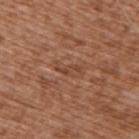follow-up — catalogued during a skin exam; not biopsied | illumination — white-light illumination | automated lesion analysis — a shape eccentricity near 0.95 and a shape-asymmetry score of about 0.5 (0 = symmetric); about 7 CIELAB-L* units darker than the surrounding skin and a lesion-to-skin contrast of about 5.5 (normalized; higher = more distinct); a border-irregularity rating of about 7.5/10 and peripheral color asymmetry of about 0; an automated nevus-likeness rating near 0 out of 100 and a lesion-detection confidence of about 65/100 | site — the upper back | acquisition — total-body-photography crop, ~15 mm field of view | subject — male, aged 73–77.